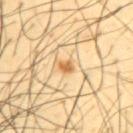{"biopsy_status": "not biopsied; imaged during a skin examination", "patient": {"sex": "male", "age_approx": 45}, "lighting": "cross-polarized", "site": "upper back", "image": {"source": "total-body photography crop", "field_of_view_mm": 15}}